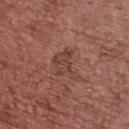biopsy status: total-body-photography surveillance lesion; no biopsy | acquisition: ~15 mm crop, total-body skin-cancer survey | subject: female, in their mid- to late 60s | lighting: white-light | location: the upper back | size: ≈4 mm.